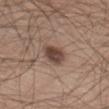Captured during whole-body skin photography for melanoma surveillance; the lesion was not biopsied. A male subject, in their 60s. From the left thigh. A 15 mm crop from a total-body photograph taken for skin-cancer surveillance.